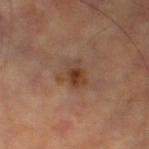Part of a total-body skin-imaging series; this lesion was reviewed on a skin check and was not flagged for biopsy.
A region of skin cropped from a whole-body photographic capture, roughly 15 mm wide.
The lesion-visualizer software estimated a lesion area of about 7 mm², a shape eccentricity near 0.25, and two-axis asymmetry of about 0.35. The software also gave a border-irregularity rating of about 4/10 and peripheral color asymmetry of about 1.5. And it measured a nevus-likeness score of about 70/100 and a lesion-detection confidence of about 100/100.
Approximately 3.5 mm at its widest.
The patient is a male aged around 65.
From the leg.
Imaged with cross-polarized lighting.